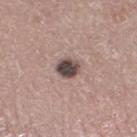This lesion was catalogued during total-body skin photography and was not selected for biopsy.
The tile uses white-light illumination.
The lesion's longest dimension is about 2.5 mm.
The lesion is located on the left lower leg.
A male patient approximately 65 years of age.
A lesion tile, about 15 mm wide, cut from a 3D total-body photograph.